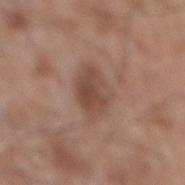Part of a total-body skin-imaging series; this lesion was reviewed on a skin check and was not flagged for biopsy. About 4.5 mm across. The lesion is located on the mid back. Cropped from a total-body skin-imaging series; the visible field is about 15 mm. Automated tile analysis of the lesion measured a lesion area of about 11 mm², an eccentricity of roughly 0.8, and a shape-asymmetry score of about 0.2 (0 = symmetric). The software also gave a mean CIELAB color near L≈47 a*≈19 b*≈26, a lesion–skin lightness drop of about 10, and a lesion-to-skin contrast of about 7 (normalized; higher = more distinct). The software also gave a border-irregularity index near 2/10, a within-lesion color-variation index near 4/10, and a peripheral color-asymmetry measure near 1.5. A male patient, in their 60s. Imaged with white-light lighting.